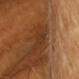This lesion was catalogued during total-body skin photography and was not selected for biopsy. From the head or neck. A roughly 15 mm field-of-view crop from a total-body skin photograph. Imaged with cross-polarized lighting. A male patient in their mid-60s. The total-body-photography lesion software estimated a lesion area of about 4.5 mm², an outline eccentricity of about 0.75 (0 = round, 1 = elongated), and a shape-asymmetry score of about 0.35 (0 = symmetric). And it measured a mean CIELAB color near L≈34 a*≈21 b*≈32 and a normalized border contrast of about 5. And it measured internal color variation of about 3 on a 0–10 scale and a peripheral color-asymmetry measure near 1.5. The lesion's longest dimension is about 3 mm.